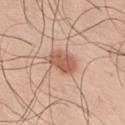| key | value |
|---|---|
| workup | catalogued during a skin exam; not biopsied |
| tile lighting | white-light illumination |
| subject | male, approximately 40 years of age |
| anatomic site | the upper back |
| image | 15 mm crop, total-body photography |